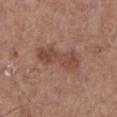Captured during whole-body skin photography for melanoma surveillance; the lesion was not biopsied.
Captured under white-light illumination.
Automated tile analysis of the lesion measured a lesion area of about 12 mm², a shape eccentricity near 0.95, and a symmetry-axis asymmetry near 0.2. The analysis additionally found a lesion color around L≈46 a*≈21 b*≈27 in CIELAB, a lesion–skin lightness drop of about 9, and a lesion-to-skin contrast of about 6.5 (normalized; higher = more distinct).
A close-up tile cropped from a whole-body skin photograph, about 15 mm across.
A male patient aged around 60.
Located on the left lower leg.
Approximately 6.5 mm at its widest.